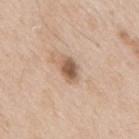Clinical impression: The lesion was photographed on a routine skin check and not biopsied; there is no pathology result. Clinical summary: This image is a 15 mm lesion crop taken from a total-body photograph. From the mid back. Measured at roughly 3 mm in maximum diameter. A male patient approximately 65 years of age. This is a white-light tile. The total-body-photography lesion software estimated a lesion area of about 5 mm², an outline eccentricity of about 0.75 (0 = round, 1 = elongated), and a symmetry-axis asymmetry near 0.25. The software also gave an average lesion color of about L≈57 a*≈18 b*≈30 (CIELAB), a lesion–skin lightness drop of about 14, and a normalized border contrast of about 9. It also reported internal color variation of about 3.5 on a 0–10 scale.